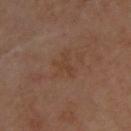follow-up: total-body-photography surveillance lesion; no biopsy | subject: male, in their mid-60s | site: the back | image source: ~15 mm crop, total-body skin-cancer survey.